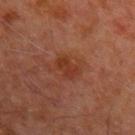Assessment: The lesion was photographed on a routine skin check and not biopsied; there is no pathology result. Clinical summary: Located on the left upper arm. A region of skin cropped from a whole-body photographic capture, roughly 15 mm wide. Automated image analysis of the tile measured an area of roughly 4.5 mm², a shape eccentricity near 0.65, and a shape-asymmetry score of about 0.2 (0 = symmetric). This is a cross-polarized tile. Approximately 2.5 mm at its widest. A male subject, aged 63 to 67.